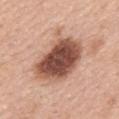Captured during whole-body skin photography for melanoma surveillance; the lesion was not biopsied. A 15 mm close-up tile from a total-body photography series done for melanoma screening. From the mid back. A female patient aged around 60. The lesion-visualizer software estimated a lesion area of about 26 mm² and a shape-asymmetry score of about 0.2 (0 = symmetric). And it measured about 19 CIELAB-L* units darker than the surrounding skin. The software also gave internal color variation of about 6.5 on a 0–10 scale and peripheral color asymmetry of about 2. Longest diameter approximately 7 mm.